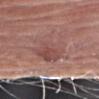No biopsy was performed on this lesion — it was imaged during a full skin examination and was not determined to be concerning.
The patient is a male in their 80s.
A 15 mm crop from a total-body photograph taken for skin-cancer surveillance.
Located on the arm.